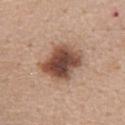The lesion was tiled from a total-body skin photograph and was not biopsied. The lesion's longest dimension is about 5 mm. Automated tile analysis of the lesion measured a mean CIELAB color near L≈48 a*≈20 b*≈27, roughly 17 lightness units darker than nearby skin, and a normalized border contrast of about 11.5. A roughly 15 mm field-of-view crop from a total-body skin photograph. The patient is a female in their mid-60s. Located on the chest. The tile uses white-light illumination.